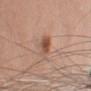workup: total-body-photography surveillance lesion; no biopsy | illumination: white-light illumination | patient: male, roughly 70 years of age | imaging modality: ~15 mm crop, total-body skin-cancer survey | site: the right upper arm | image-analysis metrics: an average lesion color of about L≈51 a*≈22 b*≈30 (CIELAB) and a lesion-to-skin contrast of about 8.5 (normalized; higher = more distinct); border irregularity of about 2 on a 0–10 scale and internal color variation of about 5 on a 0–10 scale; a nevus-likeness score of about 95/100 and a lesion-detection confidence of about 100/100.